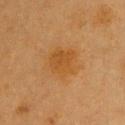{"biopsy_status": "not biopsied; imaged during a skin examination", "image": {"source": "total-body photography crop", "field_of_view_mm": 15}, "lesion_size": {"long_diameter_mm_approx": 4.5}, "automated_metrics": {"cielab_L": 44, "cielab_a": 20, "cielab_b": 39, "vs_skin_darker_L": 5.0, "border_irregularity_0_10": 2.0, "peripheral_color_asymmetry": 1.0, "lesion_detection_confidence_0_100": 100}, "lighting": "cross-polarized", "site": "upper back", "patient": {"sex": "female", "age_approx": 40}}